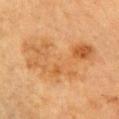Findings:
- notes · no biopsy performed (imaged during a skin exam)
- location · the right upper arm
- image source · total-body-photography crop, ~15 mm field of view
- lighting · cross-polarized
- subject · male, in their mid-80s or older
- TBP lesion metrics · an outline eccentricity of about 0.75 (0 = round, 1 = elongated) and two-axis asymmetry of about 0.4; a classifier nevus-likeness of about 0/100
- size · about 9.5 mm